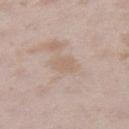Imaged during a routine full-body skin examination; the lesion was not biopsied and no histopathology is available. From the right thigh. A female subject, aged approximately 25. Measured at roughly 2.5 mm in maximum diameter. Captured under white-light illumination. An algorithmic analysis of the crop reported a lesion area of about 4.5 mm², an outline eccentricity of about 0.65 (0 = round, 1 = elongated), and a symmetry-axis asymmetry near 0.35. And it measured an average lesion color of about L≈62 a*≈15 b*≈27 (CIELAB), a lesion–skin lightness drop of about 5, and a lesion-to-skin contrast of about 4.5 (normalized; higher = more distinct). And it measured a nevus-likeness score of about 0/100 and lesion-presence confidence of about 100/100. A region of skin cropped from a whole-body photographic capture, roughly 15 mm wide.